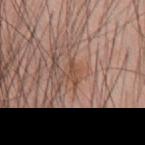No biopsy was performed on this lesion — it was imaged during a full skin examination and was not determined to be concerning.
Captured under white-light illumination.
A male patient in their 60s.
Cropped from a whole-body photographic skin survey; the tile spans about 15 mm.
The lesion is located on the mid back.
About 2.5 mm across.